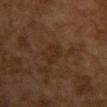Impression:
Captured during whole-body skin photography for melanoma surveillance; the lesion was not biopsied.
Image and clinical context:
The subject is a female about 60 years old. A 15 mm close-up extracted from a 3D total-body photography capture. About 3 mm across. Located on the chest. The tile uses cross-polarized illumination.